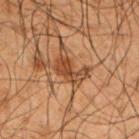Recorded during total-body skin imaging; not selected for excision or biopsy.
The lesion is on the left upper arm.
The tile uses cross-polarized illumination.
The subject is a male approximately 50 years of age.
Longest diameter approximately 4 mm.
A 15 mm close-up tile from a total-body photography series done for melanoma screening.